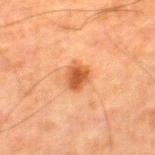{
  "biopsy_status": "not biopsied; imaged during a skin examination",
  "patient": {
    "sex": "male",
    "age_approx": 80
  },
  "image": {
    "source": "total-body photography crop",
    "field_of_view_mm": 15
  },
  "automated_metrics": {
    "nevus_likeness_0_100": 95
  },
  "lighting": "cross-polarized",
  "site": "right thigh"
}